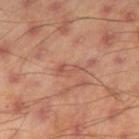This lesion was catalogued during total-body skin photography and was not selected for biopsy.
A male subject about 45 years old.
Automated tile analysis of the lesion measured a footprint of about 4 mm², an outline eccentricity of about 0.9 (0 = round, 1 = elongated), and a symmetry-axis asymmetry near 0.35. And it measured border irregularity of about 4 on a 0–10 scale and a within-lesion color-variation index near 2/10.
Located on the right thigh.
A region of skin cropped from a whole-body photographic capture, roughly 15 mm wide.